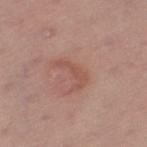follow-up: catalogued during a skin exam; not biopsied | automated metrics: a mean CIELAB color near L≈52 a*≈23 b*≈26, a lesion–skin lightness drop of about 7, and a normalized border contrast of about 5.5; a border-irregularity rating of about 7.5/10, a within-lesion color-variation index near 0.5/10, and a peripheral color-asymmetry measure near 0; lesion-presence confidence of about 100/100 | patient: female, aged 53 to 57 | location: the left thigh | imaging modality: total-body-photography crop, ~15 mm field of view | illumination: white-light.